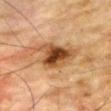The lesion-visualizer software estimated a lesion color around L≈43 a*≈20 b*≈34 in CIELAB, about 15 CIELAB-L* units darker than the surrounding skin, and a lesion-to-skin contrast of about 11 (normalized; higher = more distinct). The software also gave an automated nevus-likeness rating near 90 out of 100 and a detector confidence of about 100 out of 100 that the crop contains a lesion. The patient is a male aged approximately 85 or older. This is a cross-polarized tile. The lesion is located on the chest. A 15 mm close-up extracted from a 3D total-body photography capture.